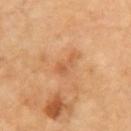  site: left upper arm
  patient:
    sex: male
    age_approx: 70
  lighting: cross-polarized
  image:
    source: total-body photography crop
    field_of_view_mm: 15